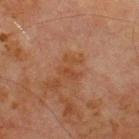Assessment: This lesion was catalogued during total-body skin photography and was not selected for biopsy. Clinical summary: Cropped from a total-body skin-imaging series; the visible field is about 15 mm. The lesion is on the chest. A male subject approximately 70 years of age.